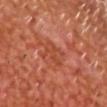Q: Was a biopsy performed?
A: imaged on a skin check; not biopsied
Q: What lighting was used for the tile?
A: cross-polarized
Q: How was this image acquired?
A: total-body-photography crop, ~15 mm field of view
Q: What are the patient's age and sex?
A: male, roughly 65 years of age